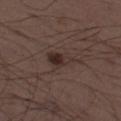Impression: The lesion was photographed on a routine skin check and not biopsied; there is no pathology result. Image and clinical context: The tile uses white-light illumination. A male subject aged approximately 50. A lesion tile, about 15 mm wide, cut from a 3D total-body photograph. On the left thigh. Longest diameter approximately 4.5 mm. An algorithmic analysis of the crop reported a lesion-to-skin contrast of about 8.5 (normalized; higher = more distinct). The software also gave a lesion-detection confidence of about 100/100.